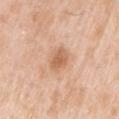Impression:
Part of a total-body skin-imaging series; this lesion was reviewed on a skin check and was not flagged for biopsy.
Background:
This is a white-light tile. Approximately 3 mm at its widest. A 15 mm close-up tile from a total-body photography series done for melanoma screening. On the left upper arm. A male subject, aged around 50.